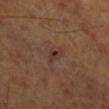Case summary:
* biopsy status — no biopsy performed (imaged during a skin exam)
* location — the leg
* image — ~15 mm crop, total-body skin-cancer survey
* image-analysis metrics — a mean CIELAB color near L≈33 a*≈18 b*≈23 and roughly 6 lightness units darker than nearby skin; a lesion-detection confidence of about 100/100
* lighting — cross-polarized
* patient — in their mid-60s
* lesion size — ~3 mm (longest diameter)